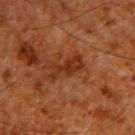Part of a total-body skin-imaging series; this lesion was reviewed on a skin check and was not flagged for biopsy. The patient is a male aged approximately 60. From the upper back. This image is a 15 mm lesion crop taken from a total-body photograph.